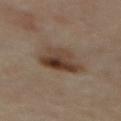Part of a total-body skin-imaging series; this lesion was reviewed on a skin check and was not flagged for biopsy.
Automated tile analysis of the lesion measured a footprint of about 15 mm² and an eccentricity of roughly 0.55. It also reported a lesion color around L≈40 a*≈15 b*≈26 in CIELAB, about 11 CIELAB-L* units darker than the surrounding skin, and a normalized lesion–skin contrast near 9.5.
A male subject aged 63–67.
The tile uses cross-polarized illumination.
From the mid back.
Measured at roughly 5 mm in maximum diameter.
A roughly 15 mm field-of-view crop from a total-body skin photograph.